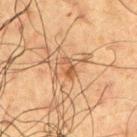Imaged during a routine full-body skin examination; the lesion was not biopsied and no histopathology is available. An algorithmic analysis of the crop reported a peripheral color-asymmetry measure near 0. The software also gave a classifier nevus-likeness of about 15/100 and a lesion-detection confidence of about 100/100. The patient is a male aged 58–62. The tile uses cross-polarized illumination. The lesion is located on the arm. This image is a 15 mm lesion crop taken from a total-body photograph. The recorded lesion diameter is about 2.5 mm.